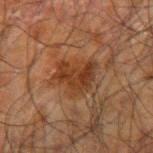Q: Is there a histopathology result?
A: imaged on a skin check; not biopsied
Q: What kind of image is this?
A: ~15 mm tile from a whole-body skin photo
Q: What is the anatomic site?
A: the right forearm
Q: Patient demographics?
A: male, roughly 70 years of age
Q: What lighting was used for the tile?
A: cross-polarized illumination
Q: How large is the lesion?
A: about 4.5 mm